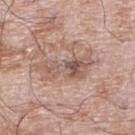<tbp_lesion>
<biopsy_status>not biopsied; imaged during a skin examination</biopsy_status>
<image>
  <source>total-body photography crop</source>
  <field_of_view_mm>15</field_of_view_mm>
</image>
<patient>
  <sex>male</sex>
  <age_approx>60</age_approx>
</patient>
<lighting>white-light</lighting>
<site>right lower leg</site>
</tbp_lesion>